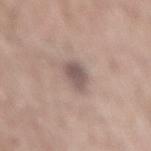Findings:
* subject — male, aged 58 to 62
* imaging modality — ~15 mm crop, total-body skin-cancer survey
* body site — the mid back
* automated lesion analysis — a lesion area of about 7 mm² and a shape-asymmetry score of about 0.2 (0 = symmetric)